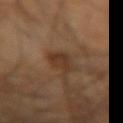Part of a total-body skin-imaging series; this lesion was reviewed on a skin check and was not flagged for biopsy. From the left upper arm. The subject is a male aged 63–67. Imaged with cross-polarized lighting. A 15 mm close-up tile from a total-body photography series done for melanoma screening.